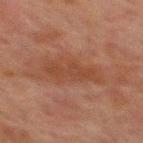biopsy status: catalogued during a skin exam; not biopsied
body site: the mid back
lesion diameter: ≈5.5 mm
image: total-body-photography crop, ~15 mm field of view
patient: male, in their 60s